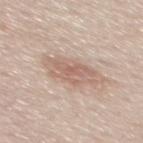Part of a total-body skin-imaging series; this lesion was reviewed on a skin check and was not flagged for biopsy. A region of skin cropped from a whole-body photographic capture, roughly 15 mm wide. The lesion is on the mid back. The patient is a male about 60 years old.